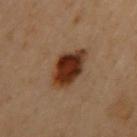{
  "biopsy_status": "not biopsied; imaged during a skin examination",
  "lighting": "cross-polarized",
  "lesion_size": {
    "long_diameter_mm_approx": 5.0
  },
  "site": "back",
  "patient": {
    "sex": "female",
    "age_approx": 60
  },
  "image": {
    "source": "total-body photography crop",
    "field_of_view_mm": 15
  }
}